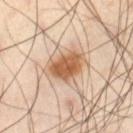Assessment:
This lesion was catalogued during total-body skin photography and was not selected for biopsy.
Context:
Located on the left thigh. A male patient, aged 53 to 57. The tile uses cross-polarized illumination. A 15 mm close-up tile from a total-body photography series done for melanoma screening.